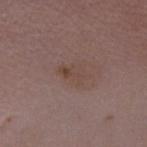follow-up=total-body-photography surveillance lesion; no biopsy
size=≈3.5 mm
anatomic site=the chest
subject=female, in their mid-30s
illumination=white-light
imaging modality=~15 mm crop, total-body skin-cancer survey